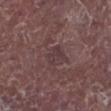Clinical impression:
This lesion was catalogued during total-body skin photography and was not selected for biopsy.
Clinical summary:
About 2.5 mm across. Automated tile analysis of the lesion measured an area of roughly 4 mm², an outline eccentricity of about 0.7 (0 = round, 1 = elongated), and a symmetry-axis asymmetry near 0.45. The software also gave a mean CIELAB color near L≈36 a*≈17 b*≈14 and a normalized lesion–skin contrast near 5.5. The analysis additionally found border irregularity of about 4.5 on a 0–10 scale, internal color variation of about 1 on a 0–10 scale, and radial color variation of about 0.5. It also reported a detector confidence of about 75 out of 100 that the crop contains a lesion. A close-up tile cropped from a whole-body skin photograph, about 15 mm across. A male patient, aged around 65. The tile uses white-light illumination. The lesion is located on the left lower leg.